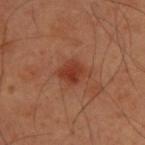Clinical impression: Imaged during a routine full-body skin examination; the lesion was not biopsied and no histopathology is available. Clinical summary: Approximately 3 mm at its widest. The patient is a male in their mid- to late 50s. This image is a 15 mm lesion crop taken from a total-body photograph. Imaged with cross-polarized lighting. The lesion is located on the upper back.